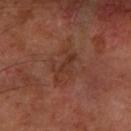<tbp_lesion>
<biopsy_status>not biopsied; imaged during a skin examination</biopsy_status>
<lighting>cross-polarized</lighting>
<lesion_size>
  <long_diameter_mm_approx>4.0</long_diameter_mm_approx>
</lesion_size>
<image>
  <source>total-body photography crop</source>
  <field_of_view_mm>15</field_of_view_mm>
</image>
<patient>
  <sex>male</sex>
  <age_approx>60</age_approx>
</patient>
<site>left forearm</site>
</tbp_lesion>